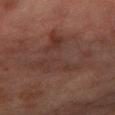Q: Was a biopsy performed?
A: catalogued during a skin exam; not biopsied
Q: What is the imaging modality?
A: 15 mm crop, total-body photography
Q: Patient demographics?
A: female, aged 53–57
Q: Automated lesion metrics?
A: an area of roughly 23 mm² and a symmetry-axis asymmetry near 0.65; a border-irregularity rating of about 10/10 and a color-variation rating of about 3.5/10; a classifier nevus-likeness of about 0/100 and a lesion-detection confidence of about 100/100
Q: Lesion location?
A: the right forearm
Q: Illumination type?
A: cross-polarized